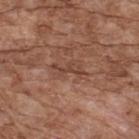Findings:
* workup — imaged on a skin check; not biopsied
* automated lesion analysis — an average lesion color of about L≈43 a*≈22 b*≈28 (CIELAB), roughly 7 lightness units darker than nearby skin, and a lesion-to-skin contrast of about 6 (normalized; higher = more distinct)
* lighting — white-light
* site — the upper back
* patient — male, aged approximately 75
* imaging modality — ~15 mm tile from a whole-body skin photo
* diameter — about 3.5 mm